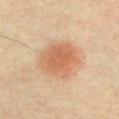The lesion was tiled from a total-body skin photograph and was not biopsied. The lesion is located on the chest. This image is a 15 mm lesion crop taken from a total-body photograph. A male patient aged 38–42.